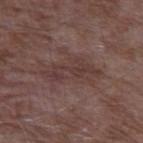Recorded during total-body skin imaging; not selected for excision or biopsy. Located on the left upper arm. An algorithmic analysis of the crop reported a footprint of about 6.5 mm², an outline eccentricity of about 0.95 (0 = round, 1 = elongated), and a shape-asymmetry score of about 0.55 (0 = symmetric). And it measured border irregularity of about 9 on a 0–10 scale, a within-lesion color-variation index near 1/10, and a peripheral color-asymmetry measure near 0. The analysis additionally found an automated nevus-likeness rating near 0 out of 100. A male subject, in their mid- to late 60s. This is a white-light tile. A 15 mm close-up extracted from a 3D total-body photography capture. Measured at roughly 6 mm in maximum diameter.